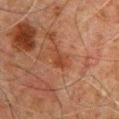  biopsy_status: not biopsied; imaged during a skin examination
  lesion_size:
    long_diameter_mm_approx: 3.0
  site: chest
  image:
    source: total-body photography crop
    field_of_view_mm: 15
  automated_metrics:
    area_mm2_approx: 3.5
    shape_asymmetry: 0.35
    border_irregularity_0_10: 3.5
    color_variation_0_10: 1.5
    peripheral_color_asymmetry: 0.5
    nevus_likeness_0_100: 0
    lesion_detection_confidence_0_100: 100
  patient:
    sex: male
    age_approx: 60
  lighting: cross-polarized